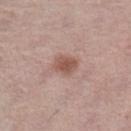Clinical impression:
The lesion was photographed on a routine skin check and not biopsied; there is no pathology result.
Acquisition and patient details:
Captured under white-light illumination. On the left lower leg. A lesion tile, about 15 mm wide, cut from a 3D total-body photograph. About 3 mm across. The patient is a female aged approximately 65.